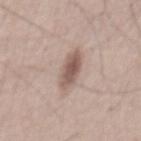biopsy_status: not biopsied; imaged during a skin examination
automated_metrics:
  vs_skin_darker_L: 12.0
  vs_skin_contrast_norm: 8.0
  border_irregularity_0_10: 3.0
  color_variation_0_10: 3.5
lighting: white-light
lesion_size:
  long_diameter_mm_approx: 5.0
patient:
  sex: male
  age_approx: 55
image:
  source: total-body photography crop
  field_of_view_mm: 15